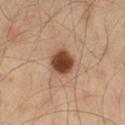{
  "biopsy_status": "not biopsied; imaged during a skin examination",
  "lesion_size": {
    "long_diameter_mm_approx": 3.0
  },
  "automated_metrics": {
    "area_mm2_approx": 7.5,
    "eccentricity": 0.4,
    "cielab_L": 39,
    "cielab_a": 20,
    "cielab_b": 29,
    "vs_skin_darker_L": 17.0,
    "vs_skin_contrast_norm": 13.0,
    "border_irregularity_0_10": 1.5,
    "color_variation_0_10": 3.5,
    "peripheral_color_asymmetry": 1.0,
    "nevus_likeness_0_100": 100,
    "lesion_detection_confidence_0_100": 100
  },
  "site": "right thigh",
  "image": {
    "source": "total-body photography crop",
    "field_of_view_mm": 15
  },
  "patient": {
    "sex": "male",
    "age_approx": 60
  }
}